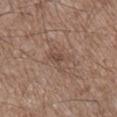Captured during whole-body skin photography for melanoma surveillance; the lesion was not biopsied.
The patient is a male aged 53 to 57.
Located on the right lower leg.
A 15 mm crop from a total-body photograph taken for skin-cancer surveillance.